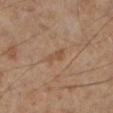  biopsy_status: not biopsied; imaged during a skin examination
  patient:
    sex: male
    age_approx: 55
  automated_metrics:
    area_mm2_approx: 3.0
    eccentricity: 0.9
    shape_asymmetry: 0.4
    nevus_likeness_0_100: 0
    lesion_detection_confidence_0_100: 100
  image:
    source: total-body photography crop
    field_of_view_mm: 15
  site: left lower leg